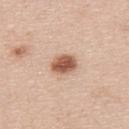This lesion was catalogued during total-body skin photography and was not selected for biopsy.
Captured under white-light illumination.
Approximately 3 mm at its widest.
From the upper back.
The patient is a male aged 43–47.
A lesion tile, about 15 mm wide, cut from a 3D total-body photograph.